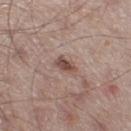The lesion was tiled from a total-body skin photograph and was not biopsied. A male subject, about 65 years old. The lesion is on the leg. This is a white-light tile. The total-body-photography lesion software estimated roughly 11 lightness units darker than nearby skin. A lesion tile, about 15 mm wide, cut from a 3D total-body photograph.